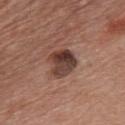follow-up — imaged on a skin check; not biopsied | location — the chest | lighting — white-light | subject — male, in their mid- to late 60s | lesion size — about 3.5 mm | image source — ~15 mm tile from a whole-body skin photo.